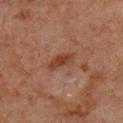Clinical impression:
This lesion was catalogued during total-body skin photography and was not selected for biopsy.
Clinical summary:
Captured under cross-polarized illumination. Measured at roughly 3.5 mm in maximum diameter. A lesion tile, about 15 mm wide, cut from a 3D total-body photograph. Automated tile analysis of the lesion measured an area of roughly 4.5 mm², a shape eccentricity near 0.9, and a symmetry-axis asymmetry near 0.25. It also reported border irregularity of about 3 on a 0–10 scale and internal color variation of about 2.5 on a 0–10 scale. And it measured a detector confidence of about 100 out of 100 that the crop contains a lesion. From the chest. The patient is a male aged 58–62.